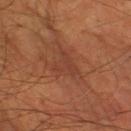Clinical impression:
Captured during whole-body skin photography for melanoma surveillance; the lesion was not biopsied.